From the left lower leg; a male subject, aged 83 or over; this image is a 15 mm lesion crop taken from a total-body photograph.
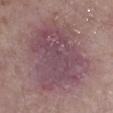histopathology: a nodular basal cell carcinoma (malignant).